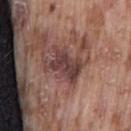Recorded during total-body skin imaging; not selected for excision or biopsy. A male subject, approximately 75 years of age. A 15 mm close-up extracted from a 3D total-body photography capture. Captured under white-light illumination. From the back. Approximately 4 mm at its widest.